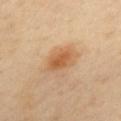biopsy status=no biopsy performed (imaged during a skin exam) | subject=female, aged 43 to 47 | body site=the mid back | lesion diameter=about 3.5 mm | image=~15 mm tile from a whole-body skin photo | illumination=cross-polarized illumination.